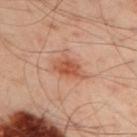biopsy status = imaged on a skin check; not biopsied
image source = ~15 mm crop, total-body skin-cancer survey
patient = male, about 50 years old
automated metrics = a shape eccentricity near 0.75 and two-axis asymmetry of about 0.3; a color-variation rating of about 3/10 and radial color variation of about 1; a classifier nevus-likeness of about 90/100 and a detector confidence of about 100 out of 100 that the crop contains a lesion
lesion size = ~4 mm (longest diameter)
lighting = cross-polarized
site = the arm